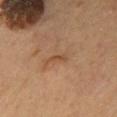Acquisition and patient details:
A 15 mm close-up extracted from a 3D total-body photography capture. Imaged with cross-polarized lighting. The lesion-visualizer software estimated roughly 7 lightness units darker than nearby skin. The lesion is on the mid back. A male patient, about 55 years old.